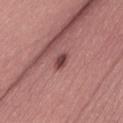Q: Is there a histopathology result?
A: no biopsy performed (imaged during a skin exam)
Q: Lesion size?
A: about 2.5 mm
Q: What lighting was used for the tile?
A: white-light illumination
Q: Who is the patient?
A: male, aged around 55
Q: What is the anatomic site?
A: the back
Q: What kind of image is this?
A: ~15 mm tile from a whole-body skin photo